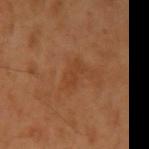Recorded during total-body skin imaging; not selected for excision or biopsy.
About 2.5 mm across.
The lesion is on the left upper arm.
A region of skin cropped from a whole-body photographic capture, roughly 15 mm wide.
Imaged with cross-polarized lighting.
A male subject, aged approximately 45.
An algorithmic analysis of the crop reported an eccentricity of roughly 0.85. The software also gave a mean CIELAB color near L≈40 a*≈23 b*≈35 and roughly 5 lightness units darker than nearby skin. It also reported a nevus-likeness score of about 0/100.